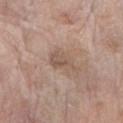Part of a total-body skin-imaging series; this lesion was reviewed on a skin check and was not flagged for biopsy. Located on the right forearm. A female subject aged approximately 85 or older. This image is a 15 mm lesion crop taken from a total-body photograph.